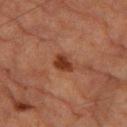| key | value |
|---|---|
| follow-up | catalogued during a skin exam; not biopsied |
| lighting | cross-polarized illumination |
| site | the left thigh |
| acquisition | ~15 mm tile from a whole-body skin photo |
| TBP lesion metrics | border irregularity of about 2 on a 0–10 scale, a within-lesion color-variation index near 2/10, and a peripheral color-asymmetry measure near 0.5; an automated nevus-likeness rating near 90 out of 100 and a lesion-detection confidence of about 100/100 |
| subject | male, approximately 85 years of age or older |
| diameter | ~2.5 mm (longest diameter) |